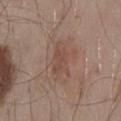Assessment: Imaged during a routine full-body skin examination; the lesion was not biopsied and no histopathology is available. Clinical summary: An algorithmic analysis of the crop reported a footprint of about 6.5 mm², an outline eccentricity of about 0.85 (0 = round, 1 = elongated), and a symmetry-axis asymmetry near 0.3. And it measured an average lesion color of about L≈47 a*≈19 b*≈25 (CIELAB), about 7 CIELAB-L* units darker than the surrounding skin, and a lesion-to-skin contrast of about 5.5 (normalized; higher = more distinct). And it measured border irregularity of about 3.5 on a 0–10 scale, a color-variation rating of about 2/10, and a peripheral color-asymmetry measure near 0.5. The software also gave a nevus-likeness score of about 0/100 and a lesion-detection confidence of about 100/100. From the mid back. A 15 mm close-up extracted from a 3D total-body photography capture. This is a white-light tile. A male patient aged 53–57.